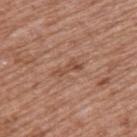Q: Was this lesion biopsied?
A: no biopsy performed (imaged during a skin exam)
Q: What is the anatomic site?
A: the upper back
Q: Illumination type?
A: white-light illumination
Q: How large is the lesion?
A: ~3.5 mm (longest diameter)
Q: What are the patient's age and sex?
A: male, about 55 years old
Q: What kind of image is this?
A: ~15 mm tile from a whole-body skin photo